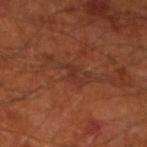Q: Was a biopsy performed?
A: imaged on a skin check; not biopsied
Q: Where on the body is the lesion?
A: the left lower leg
Q: Lesion size?
A: ~3 mm (longest diameter)
Q: Patient demographics?
A: male, aged 68 to 72
Q: How was the tile lit?
A: cross-polarized illumination
Q: What did automated image analysis measure?
A: a footprint of about 3 mm², an outline eccentricity of about 0.85 (0 = round, 1 = elongated), and two-axis asymmetry of about 0.5; a lesion color around L≈31 a*≈24 b*≈27 in CIELAB and a normalized lesion–skin contrast near 5.5; a nevus-likeness score of about 0/100 and a lesion-detection confidence of about 80/100
Q: What is the imaging modality?
A: ~15 mm crop, total-body skin-cancer survey